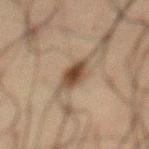Clinical impression:
Imaged during a routine full-body skin examination; the lesion was not biopsied and no histopathology is available.
Clinical summary:
This image is a 15 mm lesion crop taken from a total-body photograph. On the chest. A male patient, aged 58–62.